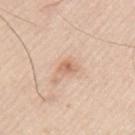The lesion was photographed on a routine skin check and not biopsied; there is no pathology result.
A male patient, aged 43–47.
A 15 mm close-up extracted from a 3D total-body photography capture.
The lesion is located on the left upper arm.
The lesion's longest dimension is about 2.5 mm.
Captured under white-light illumination.